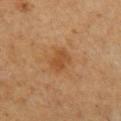Q: Was a biopsy performed?
A: imaged on a skin check; not biopsied
Q: What kind of image is this?
A: 15 mm crop, total-body photography
Q: What lighting was used for the tile?
A: cross-polarized illumination
Q: Automated lesion metrics?
A: a footprint of about 5.5 mm² and an outline eccentricity of about 0.7 (0 = round, 1 = elongated); a nevus-likeness score of about 15/100
Q: Lesion location?
A: the arm
Q: What are the patient's age and sex?
A: male, aged 58–62
Q: Lesion size?
A: ~3 mm (longest diameter)